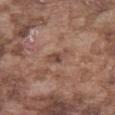{
  "biopsy_status": "not biopsied; imaged during a skin examination"
}